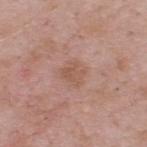The lesion was tiled from a total-body skin photograph and was not biopsied. This is a white-light tile. A male subject, aged 53–57. Longest diameter approximately 3 mm. A region of skin cropped from a whole-body photographic capture, roughly 15 mm wide. Located on the upper back.